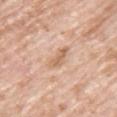Imaged during a routine full-body skin examination; the lesion was not biopsied and no histopathology is available. Cropped from a total-body skin-imaging series; the visible field is about 15 mm. The tile uses white-light illumination. A male subject, approximately 60 years of age. From the chest.